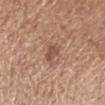biopsy status: imaged on a skin check; not biopsied | acquisition: total-body-photography crop, ~15 mm field of view | diameter: about 3 mm | location: the arm | subject: female, about 55 years old.